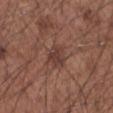No biopsy was performed on this lesion — it was imaged during a full skin examination and was not determined to be concerning. The lesion-visualizer software estimated a footprint of about 6 mm², an eccentricity of roughly 0.55, and a shape-asymmetry score of about 0.2 (0 = symmetric). It also reported border irregularity of about 2 on a 0–10 scale, internal color variation of about 2 on a 0–10 scale, and radial color variation of about 0.5. Located on the left forearm. The tile uses white-light illumination. A lesion tile, about 15 mm wide, cut from a 3D total-body photograph. A male subject, roughly 55 years of age.